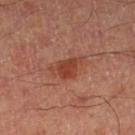  biopsy_status: not biopsied; imaged during a skin examination
  automated_metrics:
    area_mm2_approx: 6.5
    eccentricity: 0.8
    shape_asymmetry: 0.4
    border_irregularity_0_10: 4.5
  site: left lower leg
  image:
    source: total-body photography crop
    field_of_view_mm: 15
  patient:
    sex: male
    age_approx: 65
  lesion_size:
    long_diameter_mm_approx: 4.0